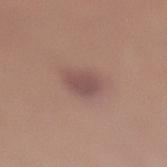The lesion was photographed on a routine skin check and not biopsied; there is no pathology result.
An algorithmic analysis of the crop reported a footprint of about 5.5 mm², an eccentricity of roughly 0.55, and a shape-asymmetry score of about 0.2 (0 = symmetric).
The patient is a female aged around 20.
This is a white-light tile.
The lesion's longest dimension is about 3 mm.
A close-up tile cropped from a whole-body skin photograph, about 15 mm across.
Located on the right lower leg.